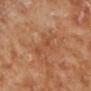biopsy status: total-body-photography surveillance lesion; no biopsy | lighting: cross-polarized illumination | anatomic site: the left lower leg | lesion diameter: about 5.5 mm | image-analysis metrics: a lesion-detection confidence of about 100/100 | patient: male, aged 68 to 72 | image source: total-body-photography crop, ~15 mm field of view.